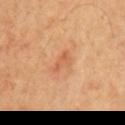Findings:
- notes — catalogued during a skin exam; not biopsied
- patient — male, approximately 70 years of age
- image source — 15 mm crop, total-body photography
- illumination — cross-polarized
- anatomic site — the chest